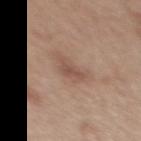Clinical impression:
No biopsy was performed on this lesion — it was imaged during a full skin examination and was not determined to be concerning.
Acquisition and patient details:
A male subject roughly 65 years of age. The recorded lesion diameter is about 2.5 mm. On the upper back. Imaged with white-light lighting. Cropped from a whole-body photographic skin survey; the tile spans about 15 mm.